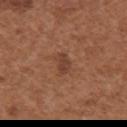<lesion>
  <biopsy_status>not biopsied; imaged during a skin examination</biopsy_status>
  <site>upper back</site>
  <lesion_size>
    <long_diameter_mm_approx>2.5</long_diameter_mm_approx>
  </lesion_size>
  <image>
    <source>total-body photography crop</source>
    <field_of_view_mm>15</field_of_view_mm>
  </image>
  <patient>
    <sex>female</sex>
    <age_approx>40</age_approx>
  </patient>
  <automated_metrics>
    <cielab_L>41</cielab_L>
    <cielab_a>22</cielab_a>
    <cielab_b>29</cielab_b>
    <border_irregularity_0_10>2.5</border_irregularity_0_10>
    <color_variation_0_10>1.0</color_variation_0_10>
    <peripheral_color_asymmetry>0.5</peripheral_color_asymmetry>
  </automated_metrics>
  <lighting>white-light</lighting>
</lesion>